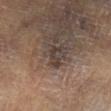Part of a total-body skin-imaging series; this lesion was reviewed on a skin check and was not flagged for biopsy. A 15 mm close-up tile from a total-body photography series done for melanoma screening. The subject is a male in their mid-60s. The lesion is on the right lower leg.